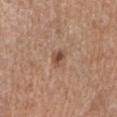Clinical impression: Imaged during a routine full-body skin examination; the lesion was not biopsied and no histopathology is available. Acquisition and patient details: A female subject, aged around 55. This image is a 15 mm lesion crop taken from a total-body photograph. The tile uses white-light illumination. The lesion is on the right forearm. Longest diameter approximately 2.5 mm.